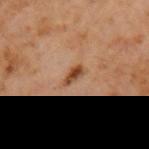The lesion was tiled from a total-body skin photograph and was not biopsied. Approximately 2.5 mm at its widest. On the left upper arm. This image is a 15 mm lesion crop taken from a total-body photograph. A male patient aged approximately 60. Automated image analysis of the tile measured a shape eccentricity near 0.9 and a symmetry-axis asymmetry near 0.25. The analysis additionally found an average lesion color of about L≈43 a*≈21 b*≈33 (CIELAB), about 13 CIELAB-L* units darker than the surrounding skin, and a normalized lesion–skin contrast near 10. The tile uses cross-polarized illumination.